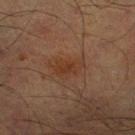* follow-up: no biopsy performed (imaged during a skin exam)
* site: the left lower leg
* subject: male, roughly 65 years of age
* automated lesion analysis: a lesion area of about 5.5 mm², a shape eccentricity near 0.8, and two-axis asymmetry of about 0.3; peripheral color asymmetry of about 0.5; a lesion-detection confidence of about 100/100
* lighting: cross-polarized
* image: total-body-photography crop, ~15 mm field of view